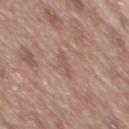Imaged during a routine full-body skin examination; the lesion was not biopsied and no histopathology is available.
Longest diameter approximately 2.5 mm.
On the mid back.
This is a white-light tile.
A lesion tile, about 15 mm wide, cut from a 3D total-body photograph.
A male patient, aged approximately 50.